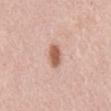  biopsy_status: not biopsied; imaged during a skin examination
  image:
    source: total-body photography crop
    field_of_view_mm: 15
  patient:
    sex: female
    age_approx: 65
  lesion_size:
    long_diameter_mm_approx: 3.0
  site: back